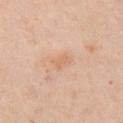Imaged during a routine full-body skin examination; the lesion was not biopsied and no histopathology is available. A male patient aged 38 to 42. Located on the arm. Approximately 2.5 mm at its widest. This image is a 15 mm lesion crop taken from a total-body photograph.